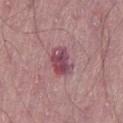follow-up — no biopsy performed (imaged during a skin exam)
diameter — ≈4 mm
image — ~15 mm tile from a whole-body skin photo
subject — male, aged approximately 65
location — the left thigh
illumination — white-light illumination